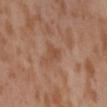The lesion was photographed on a routine skin check and not biopsied; there is no pathology result.
A female subject about 55 years old.
The recorded lesion diameter is about 3 mm.
Captured under cross-polarized illumination.
The lesion is located on the lower back.
Cropped from a total-body skin-imaging series; the visible field is about 15 mm.